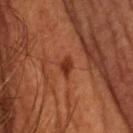subject=male, in their mid- to late 60s
acquisition=~15 mm crop, total-body skin-cancer survey
location=the chest
size=~2.5 mm (longest diameter)
lighting=cross-polarized
automated metrics=a lesion color around L≈31 a*≈27 b*≈31 in CIELAB, roughly 10 lightness units darker than nearby skin, and a normalized lesion–skin contrast near 9; border irregularity of about 2.5 on a 0–10 scale and peripheral color asymmetry of about 0.5; a lesion-detection confidence of about 100/100